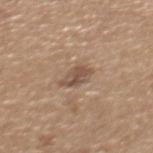Clinical impression:
The lesion was photographed on a routine skin check and not biopsied; there is no pathology result.
Context:
The lesion's longest dimension is about 3.5 mm. The tile uses white-light illumination. From the mid back. A roughly 15 mm field-of-view crop from a total-body skin photograph. The patient is a male in their mid- to late 60s.